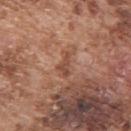The lesion was tiled from a total-body skin photograph and was not biopsied. The lesion is on the upper back. The recorded lesion diameter is about 3 mm. A 15 mm close-up tile from a total-body photography series done for melanoma screening. The tile uses white-light illumination. A male subject, about 75 years old. An algorithmic analysis of the crop reported a within-lesion color-variation index near 0/10 and peripheral color asymmetry of about 0. It also reported a nevus-likeness score of about 0/100.